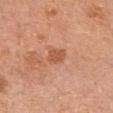{"biopsy_status": "not biopsied; imaged during a skin examination", "image": {"source": "total-body photography crop", "field_of_view_mm": 15}, "site": "head or neck", "lighting": "white-light", "lesion_size": {"long_diameter_mm_approx": 3.0}, "patient": {"sex": "female", "age_approx": 65}}